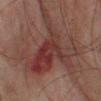Q: Is there a histopathology result?
A: catalogued during a skin exam; not biopsied
Q: What is the imaging modality?
A: 15 mm crop, total-body photography
Q: What is the anatomic site?
A: the leg
Q: Patient demographics?
A: male, aged 73 to 77
Q: How was the tile lit?
A: cross-polarized